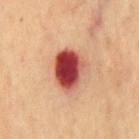notes=total-body-photography surveillance lesion; no biopsy | lighting=cross-polarized illumination | lesion size=about 5 mm | anatomic site=the front of the torso | subject=male, aged 68 to 72 | imaging modality=~15 mm tile from a whole-body skin photo.